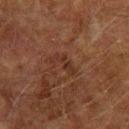<record>
  <biopsy_status>not biopsied; imaged during a skin examination</biopsy_status>
  <patient>
    <sex>male</sex>
    <age_approx>75</age_approx>
  </patient>
  <image>
    <source>total-body photography crop</source>
    <field_of_view_mm>15</field_of_view_mm>
  </image>
  <site>left upper arm</site>
</record>